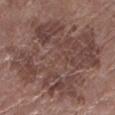| key | value |
|---|---|
| biopsy status | imaged on a skin check; not biopsied |
| tile lighting | white-light illumination |
| site | the left lower leg |
| patient | female, aged 78–82 |
| imaging modality | 15 mm crop, total-body photography |
| image-analysis metrics | a footprint of about 85 mm², an outline eccentricity of about 0.6 (0 = round, 1 = elongated), and two-axis asymmetry of about 0.2; a mean CIELAB color near L≈43 a*≈18 b*≈21 and a normalized border contrast of about 7 |
| diameter | ~13 mm (longest diameter) |